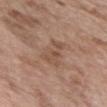Impression:
Imaged during a routine full-body skin examination; the lesion was not biopsied and no histopathology is available.
Background:
The lesion-visualizer software estimated roughly 7 lightness units darker than nearby skin and a normalized border contrast of about 5.5. A 15 mm close-up tile from a total-body photography series done for melanoma screening. The lesion's longest dimension is about 4 mm. A female subject, aged around 75. On the chest.